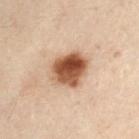Part of a total-body skin-imaging series; this lesion was reviewed on a skin check and was not flagged for biopsy. Automated tile analysis of the lesion measured a lesion color around L≈44 a*≈19 b*≈28 in CIELAB, about 17 CIELAB-L* units darker than the surrounding skin, and a normalized lesion–skin contrast near 12.5. The analysis additionally found a border-irregularity index near 2/10 and a within-lesion color-variation index near 7.5/10. The software also gave an automated nevus-likeness rating near 100 out of 100. Measured at roughly 4.5 mm in maximum diameter. Imaged with cross-polarized lighting. From the abdomen. A female patient aged approximately 55. Cropped from a whole-body photographic skin survey; the tile spans about 15 mm.